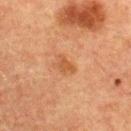Q: Was a biopsy performed?
A: total-body-photography surveillance lesion; no biopsy
Q: How large is the lesion?
A: ~2.5 mm (longest diameter)
Q: What kind of image is this?
A: ~15 mm crop, total-body skin-cancer survey
Q: What is the anatomic site?
A: the upper back
Q: Patient demographics?
A: female, roughly 55 years of age
Q: How was the tile lit?
A: cross-polarized